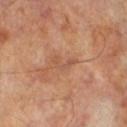| field | value |
|---|---|
| biopsy status | no biopsy performed (imaged during a skin exam) |
| body site | the left lower leg |
| image | total-body-photography crop, ~15 mm field of view |
| patient | male, roughly 70 years of age |
| lesion size | ≈3 mm |
| automated lesion analysis | a footprint of about 3.5 mm², an outline eccentricity of about 0.85 (0 = round, 1 = elongated), and a shape-asymmetry score of about 0.55 (0 = symmetric); a lesion color around L≈52 a*≈22 b*≈32 in CIELAB, about 7 CIELAB-L* units darker than the surrounding skin, and a lesion-to-skin contrast of about 4.5 (normalized; higher = more distinct); border irregularity of about 6 on a 0–10 scale, a color-variation rating of about 0/10, and radial color variation of about 0; a classifier nevus-likeness of about 0/100 and a lesion-detection confidence of about 100/100 |